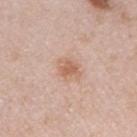Captured during whole-body skin photography for melanoma surveillance; the lesion was not biopsied.
From the arm.
The subject is a male roughly 40 years of age.
A 15 mm close-up tile from a total-body photography series done for melanoma screening.